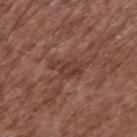Notes:
• follow-up — imaged on a skin check; not biopsied
• site — the upper back
• diameter — ~3.5 mm (longest diameter)
• imaging modality — ~15 mm crop, total-body skin-cancer survey
• automated lesion analysis — a detector confidence of about 95 out of 100 that the crop contains a lesion
• subject — male, aged approximately 75